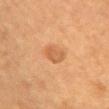{
  "biopsy_status": "not biopsied; imaged during a skin examination"
}